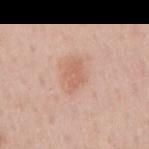The lesion was photographed on a routine skin check and not biopsied; there is no pathology result.
Located on the right upper arm.
A male subject roughly 60 years of age.
An algorithmic analysis of the crop reported an average lesion color of about L≈63 a*≈22 b*≈31 (CIELAB), a lesion–skin lightness drop of about 8, and a lesion-to-skin contrast of about 6 (normalized; higher = more distinct). And it measured a peripheral color-asymmetry measure near 0.5. The software also gave an automated nevus-likeness rating near 35 out of 100 and lesion-presence confidence of about 100/100.
Imaged with white-light lighting.
A roughly 15 mm field-of-view crop from a total-body skin photograph.